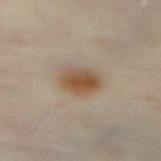| key | value |
|---|---|
| biopsy status | no biopsy performed (imaged during a skin exam) |
| subject | female, roughly 60 years of age |
| location | the abdomen |
| automated lesion analysis | a footprint of about 8.5 mm², a shape eccentricity near 0.6, and a symmetry-axis asymmetry near 0.1; an average lesion color of about L≈50 a*≈15 b*≈30 (CIELAB), about 11 CIELAB-L* units darker than the surrounding skin, and a normalized lesion–skin contrast near 9.5 |
| imaging modality | 15 mm crop, total-body photography |
| illumination | cross-polarized illumination |
| size | ≈3.5 mm |